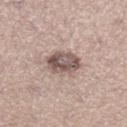Impression:
No biopsy was performed on this lesion — it was imaged during a full skin examination and was not determined to be concerning.
Background:
Cropped from a whole-body photographic skin survey; the tile spans about 15 mm. An algorithmic analysis of the crop reported an average lesion color of about L≈54 a*≈15 b*≈20 (CIELAB), about 14 CIELAB-L* units darker than the surrounding skin, and a normalized lesion–skin contrast near 9.5. This is a white-light tile. On the right lower leg. Longest diameter approximately 4 mm. A female patient, aged 33 to 37.